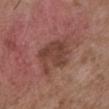Automated tile analysis of the lesion measured a border-irregularity rating of about 4.5/10 and a peripheral color-asymmetry measure near 1. It also reported a classifier nevus-likeness of about 10/100 and a detector confidence of about 100 out of 100 that the crop contains a lesion. A region of skin cropped from a whole-body photographic capture, roughly 15 mm wide. A male subject, aged around 50. Measured at roughly 5.5 mm in maximum diameter. The lesion is located on the chest.